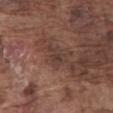Imaged during a routine full-body skin examination; the lesion was not biopsied and no histopathology is available.
A male subject approximately 75 years of age.
Located on the front of the torso.
A 15 mm crop from a total-body photograph taken for skin-cancer surveillance.
The recorded lesion diameter is about 3 mm.
The tile uses white-light illumination.